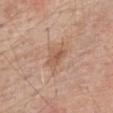Imaged during a routine full-body skin examination; the lesion was not biopsied and no histopathology is available. A 15 mm close-up extracted from a 3D total-body photography capture. A male subject, aged 78–82. On the chest. Automated tile analysis of the lesion measured a footprint of about 6.5 mm², a shape eccentricity near 0.85, and a shape-asymmetry score of about 0.4 (0 = symmetric). The software also gave a nevus-likeness score of about 0/100 and a lesion-detection confidence of about 100/100. Imaged with white-light lighting. About 4 mm across.